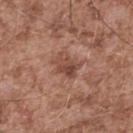Q: Was this lesion biopsied?
A: no biopsy performed (imaged during a skin exam)